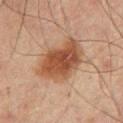A region of skin cropped from a whole-body photographic capture, roughly 15 mm wide. A male subject aged 63–67. The lesion is located on the upper back.